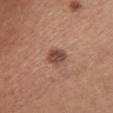biopsy status: no biopsy performed (imaged during a skin exam)
illumination: white-light
size: ~2.5 mm (longest diameter)
anatomic site: the chest
automated lesion analysis: a footprint of about 4.5 mm², an outline eccentricity of about 0.55 (0 = round, 1 = elongated), and a shape-asymmetry score of about 0.25 (0 = symmetric); border irregularity of about 2 on a 0–10 scale and a color-variation rating of about 3.5/10; a detector confidence of about 100 out of 100 that the crop contains a lesion
subject: female, roughly 45 years of age
acquisition: 15 mm crop, total-body photography